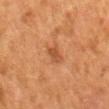<case>
  <biopsy_status>not biopsied; imaged during a skin examination</biopsy_status>
  <automated_metrics>
    <area_mm2_approx>4.0</area_mm2_approx>
    <eccentricity>0.75</eccentricity>
    <shape_asymmetry>0.25</shape_asymmetry>
    <nevus_likeness_0_100>35</nevus_likeness_0_100>
    <lesion_detection_confidence_0_100>100</lesion_detection_confidence_0_100>
  </automated_metrics>
  <site>mid back</site>
  <lesion_size>
    <long_diameter_mm_approx>2.5</long_diameter_mm_approx>
  </lesion_size>
  <image>
    <source>total-body photography crop</source>
    <field_of_view_mm>15</field_of_view_mm>
  </image>
  <lighting>cross-polarized</lighting>
  <patient>
    <sex>female</sex>
    <age_approx>50</age_approx>
  </patient>
</case>